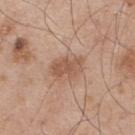{
  "biopsy_status": "not biopsied; imaged during a skin examination",
  "image": {
    "source": "total-body photography crop",
    "field_of_view_mm": 15
  },
  "patient": {
    "sex": "male",
    "age_approx": 55
  },
  "lesion_size": {
    "long_diameter_mm_approx": 3.5
  },
  "site": "upper back",
  "lighting": "white-light"
}